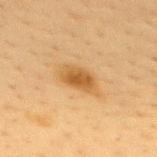Q: Was this lesion biopsied?
A: imaged on a skin check; not biopsied
Q: Lesion size?
A: about 4 mm
Q: Patient demographics?
A: female, aged 38 to 42
Q: What did automated image analysis measure?
A: a detector confidence of about 100 out of 100 that the crop contains a lesion
Q: Illumination type?
A: cross-polarized illumination
Q: What kind of image is this?
A: total-body-photography crop, ~15 mm field of view
Q: What is the anatomic site?
A: the back